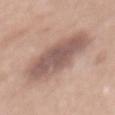Captured during whole-body skin photography for melanoma surveillance; the lesion was not biopsied. Automated tile analysis of the lesion measured an average lesion color of about L≈55 a*≈18 b*≈23 (CIELAB). The software also gave an automated nevus-likeness rating near 15 out of 100 and a detector confidence of about 100 out of 100 that the crop contains a lesion. The lesion is located on the mid back. The tile uses white-light illumination. A close-up tile cropped from a whole-body skin photograph, about 15 mm across. Longest diameter approximately 8 mm. A female patient about 40 years old.